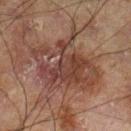workup: no biopsy performed (imaged during a skin exam) | size: about 8 mm | body site: the leg | image source: total-body-photography crop, ~15 mm field of view | automated metrics: border irregularity of about 6.5 on a 0–10 scale, internal color variation of about 5.5 on a 0–10 scale, and a peripheral color-asymmetry measure near 2; an automated nevus-likeness rating near 0 out of 100 | subject: male, aged around 60 | illumination: cross-polarized illumination.